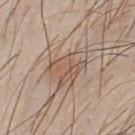Impression:
Captured during whole-body skin photography for melanoma surveillance; the lesion was not biopsied.
Clinical summary:
An algorithmic analysis of the crop reported a mean CIELAB color near L≈56 a*≈16 b*≈27, roughly 8 lightness units darker than nearby skin, and a lesion-to-skin contrast of about 6 (normalized; higher = more distinct). The lesion is located on the front of the torso. About 5 mm across. A 15 mm crop from a total-body photograph taken for skin-cancer surveillance. A male patient about 40 years old.